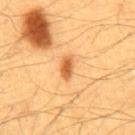Q: Is there a histopathology result?
A: imaged on a skin check; not biopsied
Q: What is the lesion's diameter?
A: ≈3 mm
Q: Who is the patient?
A: male, aged around 60
Q: What is the imaging modality?
A: 15 mm crop, total-body photography
Q: Automated lesion metrics?
A: a footprint of about 4 mm², an eccentricity of roughly 0.85, and a shape-asymmetry score of about 0.2 (0 = symmetric); a lesion color around L≈58 a*≈25 b*≈44 in CIELAB, a lesion–skin lightness drop of about 13, and a normalized lesion–skin contrast near 8.5; a nevus-likeness score of about 70/100 and a detector confidence of about 100 out of 100 that the crop contains a lesion
Q: Where on the body is the lesion?
A: the abdomen